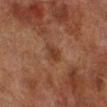Recorded during total-body skin imaging; not selected for excision or biopsy. A 15 mm close-up extracted from a 3D total-body photography capture. The lesion is on the right lower leg. The patient is a male roughly 70 years of age.